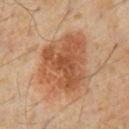  biopsy_status: not biopsied; imaged during a skin examination
  site: abdomen
  lighting: cross-polarized
  automated_metrics:
    shape_asymmetry: 0.25
    nevus_likeness_0_100: 40
  image:
    source: total-body photography crop
    field_of_view_mm: 15
  patient:
    sex: male
    age_approx: 60